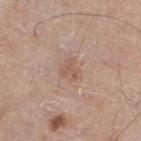- notes — imaged on a skin check; not biopsied
- body site — the right thigh
- subject — male, aged 58 to 62
- illumination — white-light illumination
- image source — ~15 mm tile from a whole-body skin photo
- size — ~2.5 mm (longest diameter)
- TBP lesion metrics — a lesion-to-skin contrast of about 5 (normalized; higher = more distinct)